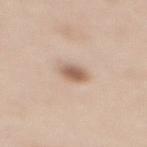Impression: Captured during whole-body skin photography for melanoma surveillance; the lesion was not biopsied. Context: The lesion is located on the mid back. About 2.5 mm across. Cropped from a whole-body photographic skin survey; the tile spans about 15 mm. A female patient aged approximately 50.